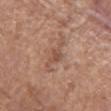Imaged during a routine full-body skin examination; the lesion was not biopsied and no histopathology is available.
A female patient, aged 63 to 67.
A lesion tile, about 15 mm wide, cut from a 3D total-body photograph.
From the right upper arm.